From the head or neck · a 15 mm close-up tile from a total-body photography series done for melanoma screening · a male patient, about 60 years old: 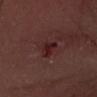lesion_size:
  long_diameter_mm_approx: 4.0
lighting: white-light
automated_metrics:
  area_mm2_approx: 5.0
  eccentricity: 0.9
  shape_asymmetry: 0.35
  cielab_L: 23
  cielab_a: 23
  cielab_b: 18
  vs_skin_darker_L: 7.0
  border_irregularity_0_10: 3.5
  color_variation_0_10: 2.0
  peripheral_color_asymmetry: 0.5
diagnosis:
  histopathology: nodular basal cell carcinoma
  malignancy: malignant
  taxonomic_path:
    - Malignant
    - Malignant adnexal epithelial proliferations - Follicular
    - Basal cell carcinoma
    - Basal cell carcinoma, Nodular